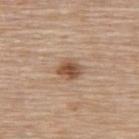Assessment: Captured during whole-body skin photography for melanoma surveillance; the lesion was not biopsied. Acquisition and patient details: The patient is a female roughly 65 years of age. Automated image analysis of the tile measured a footprint of about 5 mm² and a shape eccentricity near 0.6. The software also gave a border-irregularity index near 2/10 and internal color variation of about 4.5 on a 0–10 scale. A lesion tile, about 15 mm wide, cut from a 3D total-body photograph. This is a white-light tile. Located on the upper back. About 2.5 mm across.